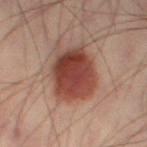Impression: Recorded during total-body skin imaging; not selected for excision or biopsy. Clinical summary: Automated image analysis of the tile measured a footprint of about 25 mm², a shape eccentricity near 0.45, and a shape-asymmetry score of about 0.15 (0 = symmetric). It also reported border irregularity of about 2 on a 0–10 scale and a peripheral color-asymmetry measure near 1.5. And it measured a detector confidence of about 100 out of 100 that the crop contains a lesion. The lesion is on the left thigh. The tile uses cross-polarized illumination. A roughly 15 mm field-of-view crop from a total-body skin photograph. The lesion's longest dimension is about 6 mm. The subject is a male aged approximately 40.